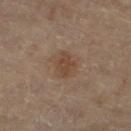biopsy status: catalogued during a skin exam; not biopsied | image: ~15 mm tile from a whole-body skin photo | anatomic site: the right thigh | subject: male, aged around 85 or older | diameter: ≈3.5 mm.